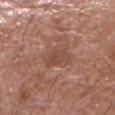A male patient aged 78 to 82.
The lesion's longest dimension is about 3.5 mm.
On the right forearm.
A close-up tile cropped from a whole-body skin photograph, about 15 mm across.
Captured under white-light illumination.